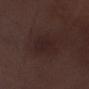This lesion was catalogued during total-body skin photography and was not selected for biopsy. A male subject, approximately 70 years of age. The lesion is located on the right lower leg. The lesion-visualizer software estimated an average lesion color of about L≈21 a*≈15 b*≈15 (CIELAB), roughly 4 lightness units darker than nearby skin, and a lesion-to-skin contrast of about 5.5 (normalized; higher = more distinct). The analysis additionally found a peripheral color-asymmetry measure near 0.5. A close-up tile cropped from a whole-body skin photograph, about 15 mm across. Measured at roughly 3.5 mm in maximum diameter.